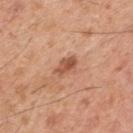Part of a total-body skin-imaging series; this lesion was reviewed on a skin check and was not flagged for biopsy. The lesion is on the mid back. Automated image analysis of the tile measured a color-variation rating of about 3.5/10 and a peripheral color-asymmetry measure near 1.5. The software also gave a classifier nevus-likeness of about 25/100 and a lesion-detection confidence of about 100/100. The recorded lesion diameter is about 3 mm. A male patient, aged approximately 45. A region of skin cropped from a whole-body photographic capture, roughly 15 mm wide.